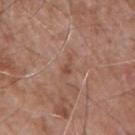Impression: This lesion was catalogued during total-body skin photography and was not selected for biopsy. Background: A male patient, approximately 75 years of age. Cropped from a whole-body photographic skin survey; the tile spans about 15 mm. The lesion is on the right upper arm.